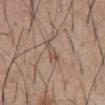notes = no biopsy performed (imaged during a skin exam)
imaging modality = ~15 mm tile from a whole-body skin photo
body site = the abdomen
size = ≈3 mm
patient = male, aged 53–57
tile lighting = white-light
TBP lesion metrics = a footprint of about 4 mm², an eccentricity of roughly 0.9, and a symmetry-axis asymmetry near 0.45; a border-irregularity rating of about 5/10, internal color variation of about 3.5 on a 0–10 scale, and a peripheral color-asymmetry measure near 1; a classifier nevus-likeness of about 0/100 and a detector confidence of about 80 out of 100 that the crop contains a lesion